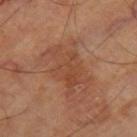Acquisition and patient details: The patient is aged around 65. From the right thigh. Measured at roughly 6 mm in maximum diameter. This image is a 15 mm lesion crop taken from a total-body photograph. The total-body-photography lesion software estimated a lesion area of about 16 mm², an eccentricity of roughly 0.8, and a symmetry-axis asymmetry near 0.4. And it measured radial color variation of about 1. Imaged with cross-polarized lighting.